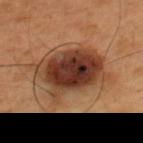No biopsy was performed on this lesion — it was imaged during a full skin examination and was not determined to be concerning.
Cropped from a total-body skin-imaging series; the visible field is about 15 mm.
On the back.
A male subject, in their 50s.
The lesion-visualizer software estimated a border-irregularity index near 3.5/10, a color-variation rating of about 8.5/10, and a peripheral color-asymmetry measure near 2.5. The software also gave an automated nevus-likeness rating near 45 out of 100 and a lesion-detection confidence of about 100/100.
The lesion's longest dimension is about 7.5 mm.